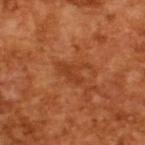| feature | finding |
|---|---|
| biopsy status | total-body-photography surveillance lesion; no biopsy |
| patient | male, in their mid-60s |
| tile lighting | cross-polarized |
| acquisition | 15 mm crop, total-body photography |